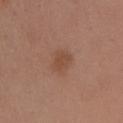| field | value |
|---|---|
| notes | catalogued during a skin exam; not biopsied |
| image source | 15 mm crop, total-body photography |
| subject | female, in their mid- to late 40s |
| illumination | white-light illumination |
| size | ~3.5 mm (longest diameter) |
| site | the right upper arm |